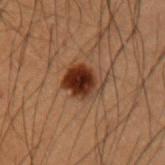The lesion was tiled from a total-body skin photograph and was not biopsied.
A male patient aged around 50.
A region of skin cropped from a whole-body photographic capture, roughly 15 mm wide.
Located on the left upper arm.